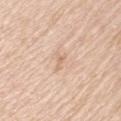A region of skin cropped from a whole-body photographic capture, roughly 15 mm wide.
The recorded lesion diameter is about 2.5 mm.
A male subject, aged around 60.
From the left upper arm.
Captured under white-light illumination.